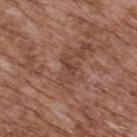follow-up: imaged on a skin check; not biopsied
site: the upper back
subject: male, aged approximately 75
image: 15 mm crop, total-body photography